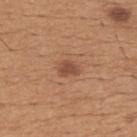Background:
The recorded lesion diameter is about 2.5 mm. A 15 mm close-up extracted from a 3D total-body photography capture. Located on the upper back. A male patient, aged approximately 65.